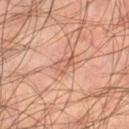{
  "patient": {
    "sex": "male",
    "age_approx": 45
  },
  "site": "right lower leg",
  "lighting": "cross-polarized",
  "image": {
    "source": "total-body photography crop",
    "field_of_view_mm": 15
  }
}